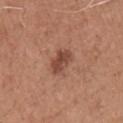Q: Was a biopsy performed?
A: total-body-photography surveillance lesion; no biopsy
Q: Where on the body is the lesion?
A: the chest
Q: How large is the lesion?
A: ≈3.5 mm
Q: Who is the patient?
A: male, roughly 65 years of age
Q: How was the tile lit?
A: white-light
Q: How was this image acquired?
A: ~15 mm tile from a whole-body skin photo